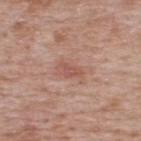Impression: Imaged during a routine full-body skin examination; the lesion was not biopsied and no histopathology is available. Acquisition and patient details: A male patient, approximately 65 years of age. From the upper back. A roughly 15 mm field-of-view crop from a total-body skin photograph.